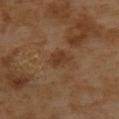Case summary:
- biopsy status — catalogued during a skin exam; not biopsied
- lighting — cross-polarized
- lesion size — about 2.5 mm
- subject — female, roughly 55 years of age
- image — ~15 mm crop, total-body skin-cancer survey
- body site — the upper back